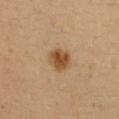No biopsy was performed on this lesion — it was imaged during a full skin examination and was not determined to be concerning. A lesion tile, about 15 mm wide, cut from a 3D total-body photograph. Captured under cross-polarized illumination. The lesion is on the chest. Automated tile analysis of the lesion measured a mean CIELAB color near L≈51 a*≈18 b*≈36, roughly 11 lightness units darker than nearby skin, and a normalized border contrast of about 9. And it measured border irregularity of about 1.5 on a 0–10 scale, a within-lesion color-variation index near 4/10, and peripheral color asymmetry of about 1.5. It also reported an automated nevus-likeness rating near 100 out of 100 and a detector confidence of about 100 out of 100 that the crop contains a lesion. The subject is a female aged around 35.